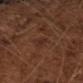biopsy status: catalogued during a skin exam; not biopsied | image source: 15 mm crop, total-body photography | diameter: ~2.5 mm (longest diameter) | TBP lesion metrics: a lesion area of about 3.5 mm² and two-axis asymmetry of about 0.25; a lesion color around L≈27 a*≈18 b*≈24 in CIELAB, about 5 CIELAB-L* units darker than the surrounding skin, and a lesion-to-skin contrast of about 5 (normalized; higher = more distinct); a border-irregularity rating of about 2.5/10 and a color-variation rating of about 2/10 | patient: male, aged approximately 65.